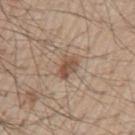Recorded during total-body skin imaging; not selected for excision or biopsy.
Captured under white-light illumination.
Measured at roughly 3 mm in maximum diameter.
The lesion is on the left upper arm.
A male subject approximately 60 years of age.
Automated tile analysis of the lesion measured a footprint of about 4.5 mm² and an eccentricity of roughly 0.75. The analysis additionally found border irregularity of about 2.5 on a 0–10 scale and a color-variation rating of about 3/10. It also reported a detector confidence of about 100 out of 100 that the crop contains a lesion.
Cropped from a whole-body photographic skin survey; the tile spans about 15 mm.